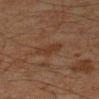Q: Is there a histopathology result?
A: imaged on a skin check; not biopsied
Q: Patient demographics?
A: male, about 45 years old
Q: What kind of image is this?
A: 15 mm crop, total-body photography
Q: Where on the body is the lesion?
A: the right forearm